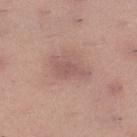biopsy_status: not biopsied; imaged during a skin examination
image:
  source: total-body photography crop
  field_of_view_mm: 15
lighting: white-light
site: left lower leg
lesion_size:
  long_diameter_mm_approx: 4.5
patient:
  sex: male
  age_approx: 35